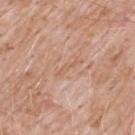A 15 mm close-up extracted from a 3D total-body photography capture.
Captured under white-light illumination.
Located on the upper back.
The patient is a male aged approximately 50.